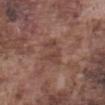biopsy_status: not biopsied; imaged during a skin examination
lighting: white-light
site: abdomen
patient:
  sex: male
  age_approx: 75
image:
  source: total-body photography crop
  field_of_view_mm: 15